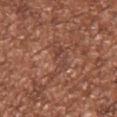This lesion was catalogued during total-body skin photography and was not selected for biopsy.
A close-up tile cropped from a whole-body skin photograph, about 15 mm across.
Automated image analysis of the tile measured a color-variation rating of about 4.5/10 and a peripheral color-asymmetry measure near 1.5. The software also gave an automated nevus-likeness rating near 0 out of 100 and a lesion-detection confidence of about 55/100.
Captured under white-light illumination.
The patient is a male about 45 years old.
From the chest.
About 5.5 mm across.